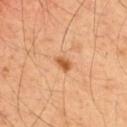biopsy status: imaged on a skin check; not biopsied
diameter: ~2 mm (longest diameter)
subject: male, aged 53–57
acquisition: ~15 mm crop, total-body skin-cancer survey
site: the back
tile lighting: cross-polarized